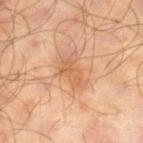Imaged during a routine full-body skin examination; the lesion was not biopsied and no histopathology is available.
The subject is a male roughly 65 years of age.
A close-up tile cropped from a whole-body skin photograph, about 15 mm across.
Located on the right lower leg.